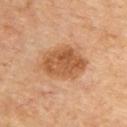Q: Was this lesion biopsied?
A: catalogued during a skin exam; not biopsied
Q: Lesion location?
A: the upper back
Q: What kind of image is this?
A: ~15 mm tile from a whole-body skin photo
Q: Illumination type?
A: cross-polarized
Q: What is the lesion's diameter?
A: about 6 mm
Q: What are the patient's age and sex?
A: male, aged 83 or older
Q: Automated lesion metrics?
A: an area of roughly 18 mm², a shape eccentricity near 0.7, and two-axis asymmetry of about 0.15; an average lesion color of about L≈54 a*≈22 b*≈37 (CIELAB) and a normalized border contrast of about 8; a color-variation rating of about 4.5/10 and peripheral color asymmetry of about 1.5